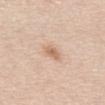| key | value |
|---|---|
| subject | male, aged 63–67 |
| size | ~2.5 mm (longest diameter) |
| anatomic site | the abdomen |
| acquisition | 15 mm crop, total-body photography |
| image-analysis metrics | a footprint of about 3.5 mm², an outline eccentricity of about 0.75 (0 = round, 1 = elongated), and a symmetry-axis asymmetry near 0.25; a border-irregularity index near 2.5/10 and a peripheral color-asymmetry measure near 0.5; an automated nevus-likeness rating near 75 out of 100 and a detector confidence of about 100 out of 100 that the crop contains a lesion |